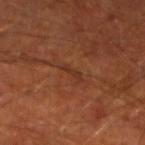Recorded during total-body skin imaging; not selected for excision or biopsy. Approximately 2.5 mm at its widest. The lesion is located on the left lower leg. This image is a 15 mm lesion crop taken from a total-body photograph. A male patient, about 70 years old.